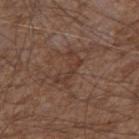Clinical impression: Imaged during a routine full-body skin examination; the lesion was not biopsied and no histopathology is available. Acquisition and patient details: Automated image analysis of the tile measured an area of roughly 6 mm² and two-axis asymmetry of about 0.5. The analysis additionally found a nevus-likeness score of about 0/100 and lesion-presence confidence of about 60/100. A male subject aged around 75. Approximately 4 mm at its widest. Imaged with white-light lighting. The lesion is located on the right upper arm. A 15 mm close-up tile from a total-body photography series done for melanoma screening.